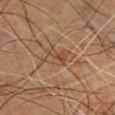Clinical impression: Captured during whole-body skin photography for melanoma surveillance; the lesion was not biopsied. Context: A 15 mm close-up extracted from a 3D total-body photography capture. About 3 mm across. Located on the chest. This is a cross-polarized tile. A male patient aged around 60. Automated tile analysis of the lesion measured a lesion area of about 5.5 mm² and two-axis asymmetry of about 0.3. It also reported a mean CIELAB color near L≈38 a*≈16 b*≈27 and a normalized border contrast of about 5. And it measured a border-irregularity index near 3.5/10, a color-variation rating of about 5.5/10, and a peripheral color-asymmetry measure near 2. The software also gave a classifier nevus-likeness of about 0/100 and lesion-presence confidence of about 95/100.